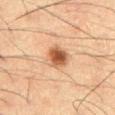This lesion was catalogued during total-body skin photography and was not selected for biopsy. The total-body-photography lesion software estimated an eccentricity of roughly 0.6 and a symmetry-axis asymmetry near 0.15. And it measured roughly 14 lightness units darker than nearby skin and a lesion-to-skin contrast of about 10 (normalized; higher = more distinct). The software also gave a nevus-likeness score of about 100/100 and a lesion-detection confidence of about 100/100. Imaged with cross-polarized lighting. Cropped from a whole-body photographic skin survey; the tile spans about 15 mm. The recorded lesion diameter is about 3.5 mm. A male subject aged approximately 60.